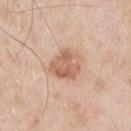biopsy_status: not biopsied; imaged during a skin examination
patient:
  sex: male
  age_approx: 55
lighting: white-light
lesion_size:
  long_diameter_mm_approx: 3.5
automated_metrics:
  border_irregularity_0_10: 2.5
  color_variation_0_10: 4.5
  peripheral_color_asymmetry: 1.5
  nevus_likeness_0_100: 15
  lesion_detection_confidence_0_100: 100
site: arm
image:
  source: total-body photography crop
  field_of_view_mm: 15